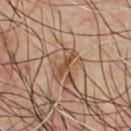Impression:
The lesion was tiled from a total-body skin photograph and was not biopsied.
Context:
Cropped from a total-body skin-imaging series; the visible field is about 15 mm. The patient is a male aged 43 to 47. This is a cross-polarized tile. The lesion is on the front of the torso. Automated image analysis of the tile measured a footprint of about 3 mm² and an outline eccentricity of about 0.95 (0 = round, 1 = elongated). And it measured a classifier nevus-likeness of about 20/100. The lesion's longest dimension is about 3 mm.